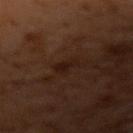Notes:
- biopsy status: total-body-photography surveillance lesion; no biopsy
- body site: the right upper arm
- illumination: cross-polarized illumination
- lesion diameter: ≈3 mm
- subject: male, aged 58 to 62
- TBP lesion metrics: a footprint of about 4 mm², a shape eccentricity near 0.8, and a shape-asymmetry score of about 0.35 (0 = symmetric); a lesion–skin lightness drop of about 4 and a normalized border contrast of about 6; internal color variation of about 1.5 on a 0–10 scale and radial color variation of about 0.5; an automated nevus-likeness rating near 0 out of 100 and a detector confidence of about 100 out of 100 that the crop contains a lesion
- acquisition: total-body-photography crop, ~15 mm field of view